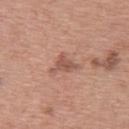This lesion was catalogued during total-body skin photography and was not selected for biopsy.
From the upper back.
About 3.5 mm across.
The patient is a female aged 58 to 62.
Automated tile analysis of the lesion measured an average lesion color of about L≈54 a*≈22 b*≈28 (CIELAB), roughly 10 lightness units darker than nearby skin, and a lesion-to-skin contrast of about 6.5 (normalized; higher = more distinct). It also reported a border-irregularity index near 5.5/10 and radial color variation of about 1.
A close-up tile cropped from a whole-body skin photograph, about 15 mm across.